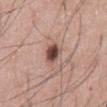  biopsy_status: not biopsied; imaged during a skin examination
  automated_metrics:
    nevus_likeness_0_100: 100
    lesion_detection_confidence_0_100: 100
  site: abdomen
  image:
    source: total-body photography crop
    field_of_view_mm: 15
  patient:
    sex: male
    age_approx: 60
  lighting: white-light